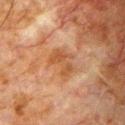| field | value |
|---|---|
| notes | imaged on a skin check; not biopsied |
| acquisition | ~15 mm crop, total-body skin-cancer survey |
| subject | male, in their 80s |
| location | the chest |